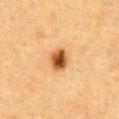biopsy_status: not biopsied; imaged during a skin examination
patient:
  sex: male
  age_approx: 85+
image:
  source: total-body photography crop
  field_of_view_mm: 15
lesion_size:
  long_diameter_mm_approx: 2.5
automated_metrics:
  color_variation_0_10: 6.5
  nevus_likeness_0_100: 100
  lesion_detection_confidence_0_100: 100
site: abdomen
lighting: cross-polarized